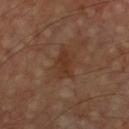No biopsy was performed on this lesion — it was imaged during a full skin examination and was not determined to be concerning.
A roughly 15 mm field-of-view crop from a total-body skin photograph.
The subject is a male aged around 60.
From the chest.